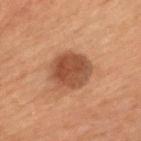notes = catalogued during a skin exam; not biopsied | imaging modality = ~15 mm crop, total-body skin-cancer survey | anatomic site = the chest | lighting = cross-polarized | subject = male, roughly 50 years of age.